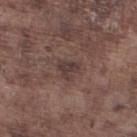| field | value |
|---|---|
| biopsy status | catalogued during a skin exam; not biopsied |
| acquisition | ~15 mm crop, total-body skin-cancer survey |
| lesion diameter | ≈2.5 mm |
| automated lesion analysis | an area of roughly 4.5 mm², a shape eccentricity near 0.55, and a shape-asymmetry score of about 0.45 (0 = symmetric); a mean CIELAB color near L≈37 a*≈16 b*≈18, roughly 8 lightness units darker than nearby skin, and a lesion-to-skin contrast of about 7 (normalized; higher = more distinct); a within-lesion color-variation index near 1.5/10 and peripheral color asymmetry of about 0.5 |
| location | the leg |
| illumination | white-light illumination |
| subject | male, roughly 75 years of age |